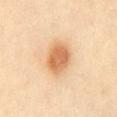A close-up tile cropped from a whole-body skin photograph, about 15 mm across.
The lesion is located on the mid back.
Measured at roughly 4.5 mm in maximum diameter.
A female patient roughly 40 years of age.